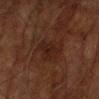notes = imaged on a skin check; not biopsied | lighting = cross-polarized illumination | lesion size = ≈4.5 mm | imaging modality = 15 mm crop, total-body photography | subject = male, aged around 75 | location = the arm.